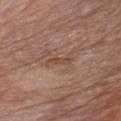Q: Was a biopsy performed?
A: total-body-photography surveillance lesion; no biopsy
Q: Patient demographics?
A: male, approximately 65 years of age
Q: What kind of image is this?
A: 15 mm crop, total-body photography
Q: Where on the body is the lesion?
A: the chest
Q: What lighting was used for the tile?
A: white-light